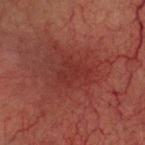{"lighting": "cross-polarized", "site": "head or neck", "lesion_size": {"long_diameter_mm_approx": 3.5}, "image": {"source": "total-body photography crop", "field_of_view_mm": 15}, "patient": {"sex": "male", "age_approx": 65}}